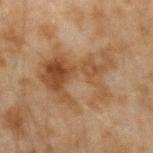This lesion was catalogued during total-body skin photography and was not selected for biopsy.
From the left forearm.
A 15 mm close-up tile from a total-body photography series done for melanoma screening.
A male patient about 45 years old.